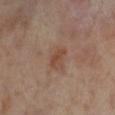Q: Was this lesion biopsied?
A: no biopsy performed (imaged during a skin exam)
Q: What are the patient's age and sex?
A: female, about 55 years old
Q: Where on the body is the lesion?
A: the leg
Q: How was this image acquired?
A: total-body-photography crop, ~15 mm field of view